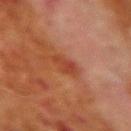Q: Was a biopsy performed?
A: no biopsy performed (imaged during a skin exam)
Q: What is the anatomic site?
A: the right upper arm
Q: How large is the lesion?
A: ≈3 mm
Q: What lighting was used for the tile?
A: cross-polarized illumination
Q: How was this image acquired?
A: total-body-photography crop, ~15 mm field of view
Q: Who is the patient?
A: male, aged approximately 70